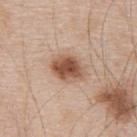No biopsy was performed on this lesion — it was imaged during a full skin examination and was not determined to be concerning.
The lesion is located on the mid back.
The recorded lesion diameter is about 4 mm.
Captured under white-light illumination.
A male patient, aged approximately 50.
Cropped from a total-body skin-imaging series; the visible field is about 15 mm.